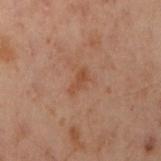No biopsy was performed on this lesion — it was imaged during a full skin examination and was not determined to be concerning. The lesion is located on the arm. Captured under cross-polarized illumination. The total-body-photography lesion software estimated an outline eccentricity of about 0.9 (0 = round, 1 = elongated) and a shape-asymmetry score of about 0.45 (0 = symmetric). And it measured a border-irregularity rating of about 4/10 and a peripheral color-asymmetry measure near 0. It also reported a classifier nevus-likeness of about 0/100 and a lesion-detection confidence of about 100/100. Approximately 3 mm at its widest. This image is a 15 mm lesion crop taken from a total-body photograph. The subject is a female aged 53 to 57.